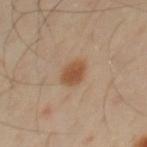This lesion was catalogued during total-body skin photography and was not selected for biopsy. Automated image analysis of the tile measured a mean CIELAB color near L≈51 a*≈19 b*≈33, about 10 CIELAB-L* units darker than the surrounding skin, and a normalized border contrast of about 8. The analysis additionally found a nevus-likeness score of about 100/100 and a detector confidence of about 100 out of 100 that the crop contains a lesion. The subject is a male aged around 40. About 3 mm across. A region of skin cropped from a whole-body photographic capture, roughly 15 mm wide. Located on the chest.